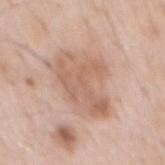| feature | finding |
|---|---|
| notes | imaged on a skin check; not biopsied |
| patient | male, aged 58 to 62 |
| acquisition | 15 mm crop, total-body photography |
| location | the mid back |
| illumination | white-light illumination |
| size | ~7 mm (longest diameter) |